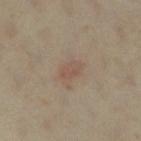follow-up = total-body-photography surveillance lesion; no biopsy | lighting = cross-polarized illumination | patient = female, approximately 35 years of age | acquisition = ~15 mm tile from a whole-body skin photo | location = the right lower leg | diameter = ≈2.5 mm.